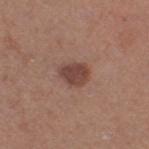follow-up: catalogued during a skin exam; not biopsied
tile lighting: white-light
image: ~15 mm tile from a whole-body skin photo
body site: the right thigh
patient: female, approximately 40 years of age
diameter: ≈3 mm